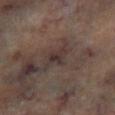The lesion was photographed on a routine skin check and not biopsied; there is no pathology result. A roughly 15 mm field-of-view crop from a total-body skin photograph. The subject is a male aged around 65. Automated image analysis of the tile measured a lesion color around L≈30 a*≈13 b*≈16 in CIELAB, roughly 7 lightness units darker than nearby skin, and a normalized border contrast of about 7.5. And it measured a border-irregularity rating of about 4/10 and a within-lesion color-variation index near 2.5/10. From the left lower leg.